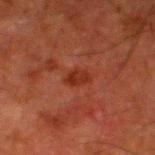The lesion was photographed on a routine skin check and not biopsied; there is no pathology result.
A male patient, aged 78 to 82.
Imaged with cross-polarized lighting.
A roughly 15 mm field-of-view crop from a total-body skin photograph.
Located on the left lower leg.